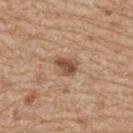workup: total-body-photography surveillance lesion; no biopsy | imaging modality: total-body-photography crop, ~15 mm field of view | patient: female, aged 68 to 72 | site: the upper back | TBP lesion metrics: a mean CIELAB color near L≈51 a*≈19 b*≈31 and a lesion–skin lightness drop of about 12; a border-irregularity index near 2.5/10, a color-variation rating of about 3.5/10, and peripheral color asymmetry of about 1.5 | illumination: white-light illumination.